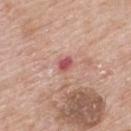| field | value |
|---|---|
| follow-up | total-body-photography surveillance lesion; no biopsy |
| acquisition | ~15 mm crop, total-body skin-cancer survey |
| location | the back |
| patient | male, aged around 65 |
| TBP lesion metrics | an area of roughly 5 mm² and an outline eccentricity of about 0.55 (0 = round, 1 = elongated); a mean CIELAB color near L≈58 a*≈26 b*≈24 and a normalized border contrast of about 6.5; a border-irregularity rating of about 4/10, a color-variation rating of about 4.5/10, and peripheral color asymmetry of about 1 |
| illumination | white-light |
| lesion size | about 3 mm |